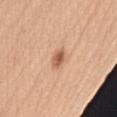Notes:
• notes — catalogued during a skin exam; not biopsied
• location — the right upper arm
• lighting — white-light
• automated metrics — a mean CIELAB color near L≈59 a*≈23 b*≈34, about 13 CIELAB-L* units darker than the surrounding skin, and a normalized border contrast of about 8; a border-irregularity index near 2.5/10, a color-variation rating of about 3.5/10, and radial color variation of about 1.5
• image source — ~15 mm crop, total-body skin-cancer survey
• patient — female, aged around 60
• lesion size — about 2.5 mm